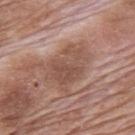follow-up — catalogued during a skin exam; not biopsied | acquisition — 15 mm crop, total-body photography | image-analysis metrics — a lesion color around L≈51 a*≈20 b*≈26 in CIELAB, a lesion–skin lightness drop of about 8, and a normalized border contrast of about 6 | lighting — white-light | anatomic site — the mid back | patient — male, in their 70s | size — ≈5.5 mm.